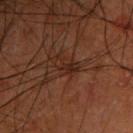| feature | finding |
|---|---|
| workup | catalogued during a skin exam; not biopsied |
| automated metrics | an eccentricity of roughly 0.95; a mean CIELAB color near L≈18 a*≈15 b*≈19, a lesion–skin lightness drop of about 5, and a normalized border contrast of about 7; a border-irregularity rating of about 4.5/10 and a peripheral color-asymmetry measure near 0; a classifier nevus-likeness of about 0/100 and a lesion-detection confidence of about 80/100 |
| diameter | about 3.5 mm |
| lighting | cross-polarized |
| body site | the front of the torso |
| image | ~15 mm crop, total-body skin-cancer survey |
| subject | male, roughly 50 years of age |